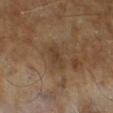Imaged during a routine full-body skin examination; the lesion was not biopsied and no histopathology is available. This is a cross-polarized tile. About 3 mm across. A 15 mm close-up tile from a total-body photography series done for melanoma screening. A male subject, aged approximately 65.